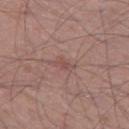biopsy_status: not biopsied; imaged during a skin examination
patient:
  sex: male
  age_approx: 55
image:
  source: total-body photography crop
  field_of_view_mm: 15
automated_metrics:
  color_variation_0_10: 0.0
  peripheral_color_asymmetry: 0.0
  nevus_likeness_0_100: 0
  lesion_detection_confidence_0_100: 100
lesion_size:
  long_diameter_mm_approx: 2.5
site: left thigh